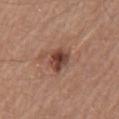{"biopsy_status": "not biopsied; imaged during a skin examination", "image": {"source": "total-body photography crop", "field_of_view_mm": 15}, "lesion_size": {"long_diameter_mm_approx": 3.0}, "lighting": "white-light", "automated_metrics": {"eccentricity": 0.5, "cielab_L": 43, "cielab_a": 22, "cielab_b": 26, "vs_skin_darker_L": 13.0, "vs_skin_contrast_norm": 9.5, "border_irregularity_0_10": 2.5, "color_variation_0_10": 5.5, "peripheral_color_asymmetry": 1.5, "nevus_likeness_0_100": 80, "lesion_detection_confidence_0_100": 100}, "patient": {"sex": "male", "age_approx": 65}, "site": "chest"}